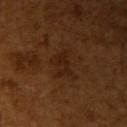Assessment:
The lesion was tiled from a total-body skin photograph and was not biopsied.
Image and clinical context:
The recorded lesion diameter is about 3 mm. A region of skin cropped from a whole-body photographic capture, roughly 15 mm wide. The tile uses cross-polarized illumination. The subject is a male approximately 65 years of age. Located on the right upper arm.